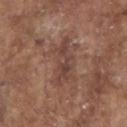Clinical impression: No biopsy was performed on this lesion — it was imaged during a full skin examination and was not determined to be concerning. Context: The total-body-photography lesion software estimated an average lesion color of about L≈44 a*≈19 b*≈24 (CIELAB), roughly 8 lightness units darker than nearby skin, and a normalized lesion–skin contrast near 6.5. The software also gave an automated nevus-likeness rating near 0 out of 100 and a lesion-detection confidence of about 90/100. The recorded lesion diameter is about 6 mm. The tile uses white-light illumination. On the head or neck. Cropped from a total-body skin-imaging series; the visible field is about 15 mm. A male patient about 80 years old.